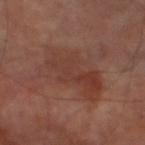Clinical impression:
Part of a total-body skin-imaging series; this lesion was reviewed on a skin check and was not flagged for biopsy.
Acquisition and patient details:
Automated tile analysis of the lesion measured a border-irregularity index near 4/10, a color-variation rating of about 5/10, and a peripheral color-asymmetry measure near 1.5. The patient is a male aged 68 to 72. A lesion tile, about 15 mm wide, cut from a 3D total-body photograph. This is a cross-polarized tile. Located on the left thigh.